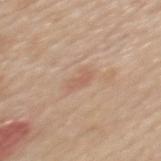Acquisition and patient details:
The lesion's longest dimension is about 2.5 mm. This is a white-light tile. This image is a 15 mm lesion crop taken from a total-body photograph. The patient is a female aged 48–52. From the upper back. The total-body-photography lesion software estimated a footprint of about 3 mm², a shape eccentricity near 0.85, and a symmetry-axis asymmetry near 0.35. It also reported a nevus-likeness score of about 0/100.